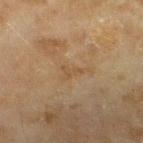A female subject, aged 58–62. An algorithmic analysis of the crop reported a lesion area of about 3 mm² and two-axis asymmetry of about 0.55. And it measured a lesion color around L≈47 a*≈14 b*≈33 in CIELAB and a normalized border contrast of about 4.5. It also reported a classifier nevus-likeness of about 0/100 and lesion-presence confidence of about 100/100. Imaged with cross-polarized lighting. This image is a 15 mm lesion crop taken from a total-body photograph. From the left lower leg. The lesion's longest dimension is about 2.5 mm.